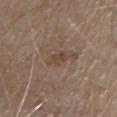Findings:
- workup · imaged on a skin check; not biopsied